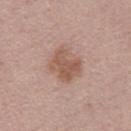Context:
A male patient aged around 30. A lesion tile, about 15 mm wide, cut from a 3D total-body photograph.